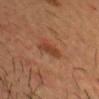<tbp_lesion>
  <biopsy_status>not biopsied; imaged during a skin examination</biopsy_status>
  <site>head or neck</site>
  <patient>
    <sex>male</sex>
    <age_approx>35</age_approx>
  </patient>
  <lesion_size>
    <long_diameter_mm_approx>3.0</long_diameter_mm_approx>
  </lesion_size>
  <image>
    <source>total-body photography crop</source>
    <field_of_view_mm>15</field_of_view_mm>
  </image>
  <automated_metrics>
    <cielab_L>38</cielab_L>
    <cielab_a>23</cielab_a>
    <cielab_b>31</cielab_b>
    <vs_skin_darker_L>8.0</vs_skin_darker_L>
    <vs_skin_contrast_norm>7.0</vs_skin_contrast_norm>
    <border_irregularity_0_10>3.5</border_irregularity_0_10>
  </automated_metrics>
  <lighting>cross-polarized</lighting>
</tbp_lesion>